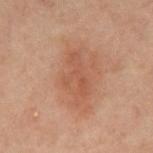biopsy status: total-body-photography surveillance lesion; no biopsy
automated lesion analysis: an outline eccentricity of about 0.9 (0 = round, 1 = elongated) and a symmetry-axis asymmetry near 0.45; a mean CIELAB color near L≈40 a*≈19 b*≈25, about 5 CIELAB-L* units darker than the surrounding skin, and a normalized border contrast of about 5; border irregularity of about 5 on a 0–10 scale and a peripheral color-asymmetry measure near 1
location: the chest
tile lighting: cross-polarized
size: ~5 mm (longest diameter)
acquisition: ~15 mm tile from a whole-body skin photo
subject: male, aged 58 to 62